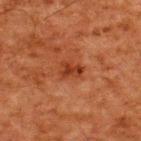workup: imaged on a skin check; not biopsied | lighting: cross-polarized | imaging modality: ~15 mm tile from a whole-body skin photo | anatomic site: the upper back | patient: male, aged 58 to 62.